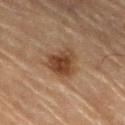<tbp_lesion>
<biopsy_status>not biopsied; imaged during a skin examination</biopsy_status>
<lesion_size>
  <long_diameter_mm_approx>4.5</long_diameter_mm_approx>
</lesion_size>
<lighting>cross-polarized</lighting>
<site>left lower leg</site>
<patient>
  <sex>male</sex>
  <age_approx>85+</age_approx>
</patient>
<image>
  <source>total-body photography crop</source>
  <field_of_view_mm>15</field_of_view_mm>
</image>
</tbp_lesion>